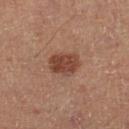No biopsy was performed on this lesion — it was imaged during a full skin examination and was not determined to be concerning. From the left lower leg. A roughly 15 mm field-of-view crop from a total-body skin photograph. This is a cross-polarized tile. The total-body-photography lesion software estimated about 10 CIELAB-L* units darker than the surrounding skin and a lesion-to-skin contrast of about 8.5 (normalized; higher = more distinct). It also reported internal color variation of about 3 on a 0–10 scale. And it measured a classifier nevus-likeness of about 85/100 and a lesion-detection confidence of about 100/100. A male patient, aged 48 to 52.